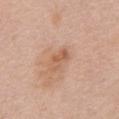Impression:
The lesion was photographed on a routine skin check and not biopsied; there is no pathology result.
Image and clinical context:
Imaged with white-light lighting. From the back. The subject is a female in their mid-60s. Measured at roughly 3 mm in maximum diameter. Cropped from a total-body skin-imaging series; the visible field is about 15 mm. An algorithmic analysis of the crop reported a lesion area of about 4.5 mm², an outline eccentricity of about 0.85 (0 = round, 1 = elongated), and a symmetry-axis asymmetry near 0.3. The analysis additionally found an automated nevus-likeness rating near 0 out of 100 and a lesion-detection confidence of about 100/100.